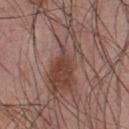Case summary:
- subject — male, about 25 years old
- location — the chest
- image source — total-body-photography crop, ~15 mm field of view